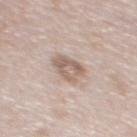Impression: Part of a total-body skin-imaging series; this lesion was reviewed on a skin check and was not flagged for biopsy. Image and clinical context: The lesion is located on the upper back. Cropped from a total-body skin-imaging series; the visible field is about 15 mm. The patient is a female aged 63–67.